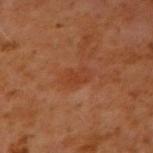patient = male, aged 58–62; lesion size = about 3.5 mm; illumination = cross-polarized illumination; image source = ~15 mm crop, total-body skin-cancer survey; anatomic site = the right upper arm.